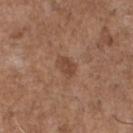This lesion was catalogued during total-body skin photography and was not selected for biopsy. A male subject aged approximately 70. The tile uses white-light illumination. Located on the chest. A close-up tile cropped from a whole-body skin photograph, about 15 mm across. The lesion-visualizer software estimated a footprint of about 4 mm², an outline eccentricity of about 0.75 (0 = round, 1 = elongated), and two-axis asymmetry of about 0.2. The software also gave a border-irregularity rating of about 2/10, internal color variation of about 1.5 on a 0–10 scale, and radial color variation of about 0.5. Longest diameter approximately 2.5 mm.